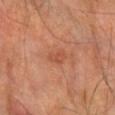The lesion was photographed on a routine skin check and not biopsied; there is no pathology result. A region of skin cropped from a whole-body photographic capture, roughly 15 mm wide. About 2.5 mm across. The lesion-visualizer software estimated a lesion area of about 3 mm², an eccentricity of roughly 0.85, and a shape-asymmetry score of about 0.4 (0 = symmetric). And it measured border irregularity of about 3.5 on a 0–10 scale, a color-variation rating of about 1/10, and peripheral color asymmetry of about 0.5. And it measured an automated nevus-likeness rating near 0 out of 100. The lesion is located on the left forearm. The patient is a male in their 70s.